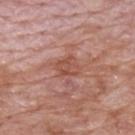follow-up: imaged on a skin check; not biopsied
location: the back
subject: male, aged 68 to 72
image-analysis metrics: a classifier nevus-likeness of about 0/100 and a lesion-detection confidence of about 100/100
lighting: white-light
diameter: ~3 mm (longest diameter)
image: total-body-photography crop, ~15 mm field of view